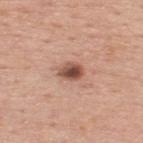follow-up — total-body-photography surveillance lesion; no biopsy | patient — male, aged around 65 | site — the upper back | image-analysis metrics — a footprint of about 5 mm² and a shape-asymmetry score of about 0.15 (0 = symmetric); a classifier nevus-likeness of about 95/100 | size — ≈3 mm | tile lighting — white-light illumination | acquisition — total-body-photography crop, ~15 mm field of view.